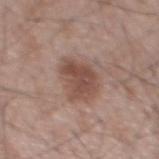site=the chest
lesion size=about 4.5 mm
tile lighting=white-light illumination
automated metrics=an area of roughly 12 mm², an eccentricity of roughly 0.55, and two-axis asymmetry of about 0.3; an automated nevus-likeness rating near 40 out of 100 and a lesion-detection confidence of about 100/100
patient=male, aged 63 to 67
acquisition=15 mm crop, total-body photography